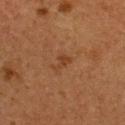workup: catalogued during a skin exam; not biopsied
image source: total-body-photography crop, ~15 mm field of view
diameter: ≈3 mm
patient: female, roughly 40 years of age
tile lighting: cross-polarized illumination
anatomic site: the upper back